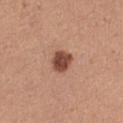Assessment: No biopsy was performed on this lesion — it was imaged during a full skin examination and was not determined to be concerning. Background: Automated image analysis of the tile measured an outline eccentricity of about 0.55 (0 = round, 1 = elongated) and a shape-asymmetry score of about 0.15 (0 = symmetric). It also reported a lesion color around L≈47 a*≈23 b*≈28 in CIELAB and a lesion–skin lightness drop of about 16. And it measured border irregularity of about 1.5 on a 0–10 scale, a within-lesion color-variation index near 3/10, and radial color variation of about 1. And it measured a classifier nevus-likeness of about 85/100 and lesion-presence confidence of about 100/100. Approximately 3 mm at its widest. The patient is a female aged 33 to 37. A region of skin cropped from a whole-body photographic capture, roughly 15 mm wide. Located on the left thigh. The tile uses white-light illumination.